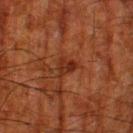Acquisition and patient details:
This is a cross-polarized tile. A 15 mm close-up tile from a total-body photography series done for melanoma screening. A male patient, about 80 years old. On the leg.